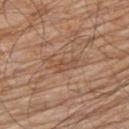No biopsy was performed on this lesion — it was imaged during a full skin examination and was not determined to be concerning. A male patient, in their 80s. A roughly 15 mm field-of-view crop from a total-body skin photograph. The lesion is on the upper back. Imaged with white-light lighting.